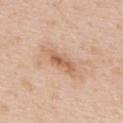The lesion was tiled from a total-body skin photograph and was not biopsied.
From the back.
A 15 mm crop from a total-body photograph taken for skin-cancer surveillance.
The lesion-visualizer software estimated an area of roughly 9 mm², an eccentricity of roughly 0.95, and a shape-asymmetry score of about 0.25 (0 = symmetric). And it measured a detector confidence of about 100 out of 100 that the crop contains a lesion.
Imaged with white-light lighting.
A male subject about 50 years old.
Approximately 6.5 mm at its widest.